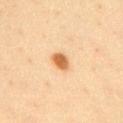  biopsy_status: not biopsied; imaged during a skin examination
  lesion_size:
    long_diameter_mm_approx: 2.5
  patient:
    sex: female
    age_approx: 50
  lighting: cross-polarized
  image:
    source: total-body photography crop
    field_of_view_mm: 15
  site: chest